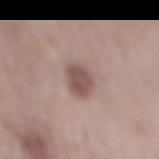workup: total-body-photography surveillance lesion; no biopsy | patient: male, aged approximately 65 | lesion size: ≈3 mm | illumination: white-light illumination | site: the mid back | imaging modality: ~15 mm tile from a whole-body skin photo.